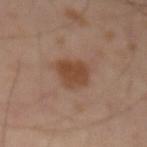Q: How was the tile lit?
A: cross-polarized
Q: Lesion size?
A: about 3.5 mm
Q: Patient demographics?
A: male, in their mid- to late 60s
Q: What did automated image analysis measure?
A: a nevus-likeness score of about 90/100 and a lesion-detection confidence of about 100/100
Q: How was this image acquired?
A: ~15 mm crop, total-body skin-cancer survey